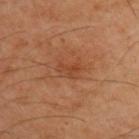{
  "biopsy_status": "not biopsied; imaged during a skin examination",
  "automated_metrics": {
    "vs_skin_darker_L": 6.0,
    "vs_skin_contrast_norm": 5.5,
    "border_irregularity_0_10": 4.0,
    "color_variation_0_10": 2.0,
    "peripheral_color_asymmetry": 0.5,
    "nevus_likeness_0_100": 0,
    "lesion_detection_confidence_0_100": 100
  },
  "lighting": "cross-polarized",
  "image": {
    "source": "total-body photography crop",
    "field_of_view_mm": 15
  },
  "site": "upper back",
  "lesion_size": {
    "long_diameter_mm_approx": 3.0
  },
  "patient": {
    "sex": "male",
    "age_approx": 50
  }
}